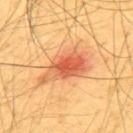The lesion was photographed on a routine skin check and not biopsied; there is no pathology result.